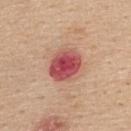Clinical impression:
Imaged during a routine full-body skin examination; the lesion was not biopsied and no histopathology is available.
Image and clinical context:
Imaged with white-light lighting. Cropped from a whole-body photographic skin survey; the tile spans about 15 mm. Measured at roughly 4 mm in maximum diameter. The patient is a female aged 43 to 47. The lesion is on the upper back.